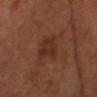The lesion was tiled from a total-body skin photograph and was not biopsied. This image is a 15 mm lesion crop taken from a total-body photograph. The tile uses cross-polarized illumination. The lesion is on the head or neck. Approximately 3.5 mm at its widest. Automated image analysis of the tile measured a footprint of about 7.5 mm², an outline eccentricity of about 0.6 (0 = round, 1 = elongated), and two-axis asymmetry of about 0.4. It also reported a nevus-likeness score of about 0/100. A male subject, in their 70s.